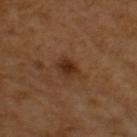| key | value |
|---|---|
| subject | female, aged 53 to 57 |
| location | the right upper arm |
| lesion size | about 3.5 mm |
| image | total-body-photography crop, ~15 mm field of view |
| lighting | cross-polarized |
| automated metrics | a shape eccentricity near 0.75 and a symmetry-axis asymmetry near 0.15; a border-irregularity rating of about 2/10 and a within-lesion color-variation index near 4/10; an automated nevus-likeness rating near 70 out of 100 |